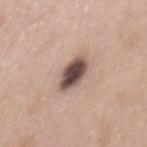workup — catalogued during a skin exam; not biopsied
size — about 4 mm
image source — 15 mm crop, total-body photography
illumination — white-light
patient — female, aged 38–42
body site — the mid back